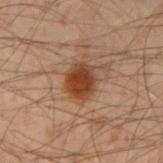biopsy status = total-body-photography surveillance lesion; no biopsy
imaging modality = 15 mm crop, total-body photography
size = ≈3.5 mm
site = the left forearm
image-analysis metrics = an average lesion color of about L≈34 a*≈20 b*≈28 (CIELAB), a lesion–skin lightness drop of about 11, and a normalized lesion–skin contrast near 10.5; a within-lesion color-variation index near 3/10 and peripheral color asymmetry of about 1; a lesion-detection confidence of about 100/100
tile lighting = cross-polarized illumination
subject = male, roughly 50 years of age